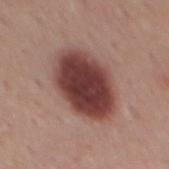| field | value |
|---|---|
| follow-up | imaged on a skin check; not biopsied |
| lighting | white-light |
| subject | male, approximately 55 years of age |
| image source | ~15 mm crop, total-body skin-cancer survey |
| body site | the back |
| diameter | ≈7.5 mm |